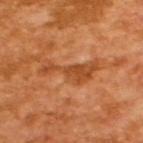Recorded during total-body skin imaging; not selected for excision or biopsy.
A male patient aged 63–67.
Imaged with cross-polarized lighting.
A lesion tile, about 15 mm wide, cut from a 3D total-body photograph.
The total-body-photography lesion software estimated a footprint of about 9.5 mm² and two-axis asymmetry of about 0.65. The analysis additionally found a border-irregularity index near 8.5/10, a within-lesion color-variation index near 2.5/10, and a peripheral color-asymmetry measure near 1. The analysis additionally found an automated nevus-likeness rating near 0 out of 100 and a detector confidence of about 100 out of 100 that the crop contains a lesion.
About 6 mm across.